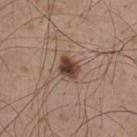Notes:
- biopsy status — imaged on a skin check; not biopsied